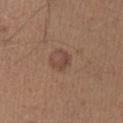Assessment:
Imaged during a routine full-body skin examination; the lesion was not biopsied and no histopathology is available.
Image and clinical context:
The tile uses white-light illumination. A male patient, aged 58–62. The lesion is on the left lower leg. The recorded lesion diameter is about 2.5 mm. A 15 mm close-up tile from a total-body photography series done for melanoma screening.